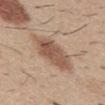The lesion's longest dimension is about 7 mm. The subject is a male roughly 30 years of age. The lesion is located on the abdomen. A lesion tile, about 15 mm wide, cut from a 3D total-body photograph. This is a white-light tile. The total-body-photography lesion software estimated a footprint of about 16 mm², an eccentricity of roughly 0.9, and a shape-asymmetry score of about 0.15 (0 = symmetric). The analysis additionally found a classifier nevus-likeness of about 90/100 and a lesion-detection confidence of about 100/100.